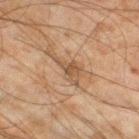<lesion>
<biopsy_status>not biopsied; imaged during a skin examination</biopsy_status>
<site>leg</site>
<patient>
  <sex>male</sex>
  <age_approx>45</age_approx>
</patient>
<lighting>cross-polarized</lighting>
<image>
  <source>total-body photography crop</source>
  <field_of_view_mm>15</field_of_view_mm>
</image>
<lesion_size>
  <long_diameter_mm_approx>4.0</long_diameter_mm_approx>
</lesion_size>
</lesion>